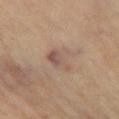| feature | finding |
|---|---|
| tile lighting | cross-polarized |
| patient | female, roughly 70 years of age |
| lesion diameter | ~3.5 mm (longest diameter) |
| site | the leg |
| image | total-body-photography crop, ~15 mm field of view |